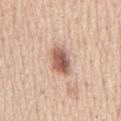• patient: male, aged 58 to 62
• body site: the mid back
• lesion diameter: ~4 mm (longest diameter)
• image source: ~15 mm crop, total-body skin-cancer survey
• tile lighting: white-light illumination